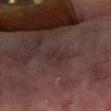– follow-up: catalogued during a skin exam; not biopsied
– image: total-body-photography crop, ~15 mm field of view
– size: ~2.5 mm (longest diameter)
– site: the left forearm
– automated metrics: a normalized border contrast of about 5.5; a classifier nevus-likeness of about 0/100
– subject: male, in their mid- to late 70s